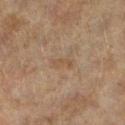subject: female, roughly 60 years of age; site: the left lower leg; lesion diameter: about 3 mm; image source: ~15 mm crop, total-body skin-cancer survey.